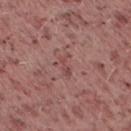Q: Was this lesion biopsied?
A: catalogued during a skin exam; not biopsied
Q: What is the imaging modality?
A: total-body-photography crop, ~15 mm field of view
Q: What lighting was used for the tile?
A: white-light
Q: Where on the body is the lesion?
A: the right thigh
Q: Who is the patient?
A: female, roughly 20 years of age
Q: How large is the lesion?
A: ~3 mm (longest diameter)